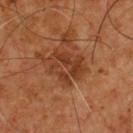follow-up: no biopsy performed (imaged during a skin exam)
imaging modality: total-body-photography crop, ~15 mm field of view
subject: male, in their mid-50s
site: the chest
diameter: ~4.5 mm (longest diameter)
lighting: cross-polarized